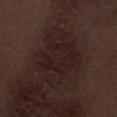* biopsy status · no biopsy performed (imaged during a skin exam)
* tile lighting · white-light
* site · the leg
* imaging modality · ~15 mm crop, total-body skin-cancer survey
* diameter · ~7 mm (longest diameter)
* patient · male, aged around 70